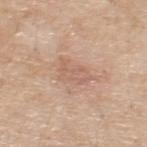* follow-up: catalogued during a skin exam; not biopsied
* automated lesion analysis: an eccentricity of roughly 0.8; a mean CIELAB color near L≈61 a*≈19 b*≈28, about 7 CIELAB-L* units darker than the surrounding skin, and a normalized border contrast of about 4.5; a nevus-likeness score of about 0/100 and a lesion-detection confidence of about 100/100
* size: ~4.5 mm (longest diameter)
* patient: male, in their 70s
* image: 15 mm crop, total-body photography
* illumination: white-light
* anatomic site: the upper back